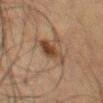{"biopsy_status": "not biopsied; imaged during a skin examination", "automated_metrics": {"shape_asymmetry": 0.3}, "lighting": "cross-polarized", "patient": {"sex": "male", "age_approx": 50}, "image": {"source": "total-body photography crop", "field_of_view_mm": 15}, "site": "chest", "lesion_size": {"long_diameter_mm_approx": 4.5}}